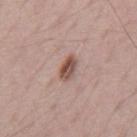Notes:
- notes · catalogued during a skin exam; not biopsied
- patient · male, aged approximately 70
- lighting · white-light illumination
- image-analysis metrics · a footprint of about 4.5 mm², a shape eccentricity near 0.85, and a shape-asymmetry score of about 0.2 (0 = symmetric); a border-irregularity rating of about 2/10 and peripheral color asymmetry of about 2
- acquisition · 15 mm crop, total-body photography
- body site · the back
- diameter · about 3 mm